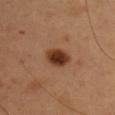| field | value |
|---|---|
| follow-up | catalogued during a skin exam; not biopsied |
| image-analysis metrics | a border-irregularity rating of about 1.5/10, a within-lesion color-variation index near 4/10, and peripheral color asymmetry of about 1; lesion-presence confidence of about 100/100 |
| site | the arm |
| size | about 3 mm |
| subject | male, aged around 50 |
| acquisition | ~15 mm crop, total-body skin-cancer survey |